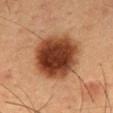{"biopsy_status": "not biopsied; imaged during a skin examination", "image": {"source": "total-body photography crop", "field_of_view_mm": 15}, "patient": {"sex": "male", "age_approx": 55}, "lesion_size": {"long_diameter_mm_approx": 7.0}, "lighting": "cross-polarized", "site": "chest"}